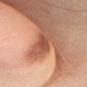The lesion was tiled from a total-body skin photograph and was not biopsied.
This is a white-light tile.
This image is a 15 mm lesion crop taken from a total-body photograph.
The patient is a female aged around 45.
Longest diameter approximately 2.5 mm.
From the head or neck.